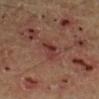{"biopsy_status": "not biopsied; imaged during a skin examination", "site": "right lower leg", "lesion_size": {"long_diameter_mm_approx": 4.0}, "patient": {"sex": "male", "age_approx": 55}, "automated_metrics": {"cielab_L": 35, "cielab_a": 24, "cielab_b": 24, "vs_skin_darker_L": 8.0, "vs_skin_contrast_norm": 7.0, "color_variation_0_10": 5.0, "peripheral_color_asymmetry": 2.0, "nevus_likeness_0_100": 0, "lesion_detection_confidence_0_100": 85}, "image": {"source": "total-body photography crop", "field_of_view_mm": 15}}